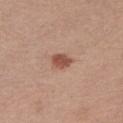Clinical impression: This lesion was catalogued during total-body skin photography and was not selected for biopsy. Acquisition and patient details: On the left upper arm. A male patient, approximately 25 years of age. The tile uses white-light illumination. A lesion tile, about 15 mm wide, cut from a 3D total-body photograph.